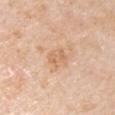Assessment:
Recorded during total-body skin imaging; not selected for excision or biopsy.
Background:
The tile uses white-light illumination. The subject is a male in their mid- to late 70s. A roughly 15 mm field-of-view crop from a total-body skin photograph. Longest diameter approximately 2.5 mm. The lesion is on the right upper arm.